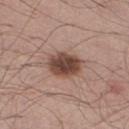Assessment:
Captured during whole-body skin photography for melanoma surveillance; the lesion was not biopsied.
Clinical summary:
Measured at roughly 4 mm in maximum diameter. A 15 mm close-up extracted from a 3D total-body photography capture. Automated tile analysis of the lesion measured a normalized lesion–skin contrast near 11. The analysis additionally found a border-irregularity rating of about 1.5/10, a within-lesion color-variation index near 5/10, and peripheral color asymmetry of about 1.5. The software also gave a nevus-likeness score of about 90/100 and lesion-presence confidence of about 100/100. A male patient, roughly 40 years of age. Located on the right thigh.